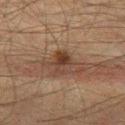Findings:
– notes: imaged on a skin check; not biopsied
– patient: male, in their 50s
– lesion size: ~3.5 mm (longest diameter)
– tile lighting: cross-polarized illumination
– location: the left thigh
– image: ~15 mm tile from a whole-body skin photo